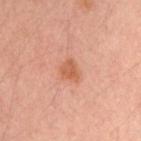The lesion was tiled from a total-body skin photograph and was not biopsied.
Longest diameter approximately 3 mm.
The subject is a male aged around 35.
From the left arm.
This image is a 15 mm lesion crop taken from a total-body photograph.